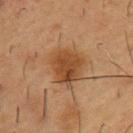This lesion was catalogued during total-body skin photography and was not selected for biopsy.
The subject is a male roughly 55 years of age.
The lesion's longest dimension is about 4.5 mm.
Cropped from a total-body skin-imaging series; the visible field is about 15 mm.
On the chest.
Imaged with cross-polarized lighting.